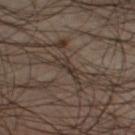Clinical impression:
Part of a total-body skin-imaging series; this lesion was reviewed on a skin check and was not flagged for biopsy.
Image and clinical context:
From the left lower leg. A male subject, aged approximately 45. A 15 mm close-up tile from a total-body photography series done for melanoma screening. An algorithmic analysis of the crop reported roughly 6 lightness units darker than nearby skin and a normalized lesion–skin contrast near 6.5. And it measured an automated nevus-likeness rating near 0 out of 100 and lesion-presence confidence of about 0/100. The tile uses cross-polarized illumination.